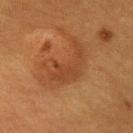Captured during whole-body skin photography for melanoma surveillance; the lesion was not biopsied.
Located on the arm.
The subject is a female in their mid-50s.
The lesion's longest dimension is about 5.5 mm.
A roughly 15 mm field-of-view crop from a total-body skin photograph.
Captured under cross-polarized illumination.